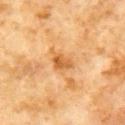Clinical impression: The lesion was tiled from a total-body skin photograph and was not biopsied. Background: A 15 mm close-up tile from a total-body photography series done for melanoma screening. The tile uses cross-polarized illumination. The subject is a male aged approximately 60. Approximately 2.5 mm at its widest. From the chest. An algorithmic analysis of the crop reported an area of roughly 4 mm² and a shape-asymmetry score of about 0.3 (0 = symmetric). The analysis additionally found a nevus-likeness score of about 5/100 and a detector confidence of about 100 out of 100 that the crop contains a lesion.